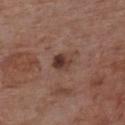The lesion was photographed on a routine skin check and not biopsied; there is no pathology result.
A male subject, roughly 60 years of age.
Cropped from a whole-body photographic skin survey; the tile spans about 15 mm.
This is a white-light tile.
Approximately 2.5 mm at its widest.
Automated image analysis of the tile measured a lesion area of about 4.5 mm² and an outline eccentricity of about 0.55 (0 = round, 1 = elongated). The analysis additionally found a border-irregularity index near 1.5/10, a within-lesion color-variation index near 6.5/10, and a peripheral color-asymmetry measure near 2.
From the chest.